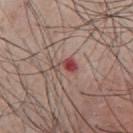biopsy_status: not biopsied; imaged during a skin examination
image:
  source: total-body photography crop
  field_of_view_mm: 15
lesion_size:
  long_diameter_mm_approx: 3.0
site: chest
lighting: white-light
patient:
  sex: male
  age_approx: 60